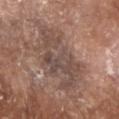Captured during whole-body skin photography for melanoma surveillance; the lesion was not biopsied. Cropped from a whole-body photographic skin survey; the tile spans about 15 mm. This is a white-light tile. Located on the head or neck. Measured at roughly 7.5 mm in maximum diameter. A male patient approximately 80 years of age.